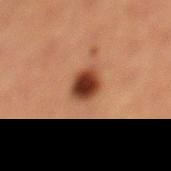{
  "biopsy_status": "not biopsied; imaged during a skin examination",
  "site": "left lower leg",
  "patient": {
    "sex": "female",
    "age_approx": 40
  },
  "lighting": "cross-polarized",
  "image": {
    "source": "total-body photography crop",
    "field_of_view_mm": 15
  },
  "automated_metrics": {
    "border_irregularity_0_10": 1.5,
    "color_variation_0_10": 5.0,
    "peripheral_color_asymmetry": 1.0
  },
  "lesion_size": {
    "long_diameter_mm_approx": 3.0
  }
}